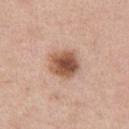follow-up: total-body-photography surveillance lesion; no biopsy | location: the left upper arm | patient: male, about 60 years old | acquisition: total-body-photography crop, ~15 mm field of view | diameter: ≈3.5 mm | image-analysis metrics: a footprint of about 10 mm² and an outline eccentricity of about 0.3 (0 = round, 1 = elongated); a classifier nevus-likeness of about 95/100 and lesion-presence confidence of about 100/100.